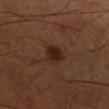Notes:
- site · the right lower leg
- diameter · about 2.5 mm
- patient · male, in their 50s
- image source · ~15 mm crop, total-body skin-cancer survey
- lighting · cross-polarized illumination